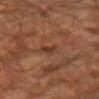Clinical impression: This lesion was catalogued during total-body skin photography and was not selected for biopsy. Acquisition and patient details: About 3 mm across. This image is a 15 mm lesion crop taken from a total-body photograph. From the right upper arm. A male patient aged around 65.